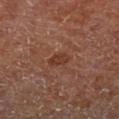{
  "biopsy_status": "not biopsied; imaged during a skin examination"
}